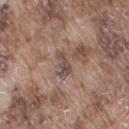No biopsy was performed on this lesion — it was imaged during a full skin examination and was not determined to be concerning. A male subject, aged around 75. The recorded lesion diameter is about 3 mm. Located on the right thigh. Cropped from a whole-body photographic skin survey; the tile spans about 15 mm. Imaged with white-light lighting.